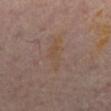Impression: Captured during whole-body skin photography for melanoma surveillance; the lesion was not biopsied. Acquisition and patient details: Automated image analysis of the tile measured a footprint of about 4.5 mm², a shape eccentricity near 0.95, and two-axis asymmetry of about 0.45. The software also gave a lesion color around L≈46 a*≈15 b*≈26 in CIELAB. The software also gave a nevus-likeness score of about 0/100 and lesion-presence confidence of about 100/100. The tile uses cross-polarized illumination. From the left leg. A male subject aged approximately 50. Longest diameter approximately 4.5 mm. A lesion tile, about 15 mm wide, cut from a 3D total-body photograph.